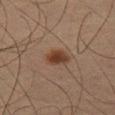Case summary:
- biopsy status · no biopsy performed (imaged during a skin exam)
- automated lesion analysis · a footprint of about 5 mm², a shape eccentricity near 0.65, and two-axis asymmetry of about 0.25; a border-irregularity rating of about 2/10, a color-variation rating of about 3.5/10, and peripheral color asymmetry of about 1; an automated nevus-likeness rating near 100 out of 100 and a lesion-detection confidence of about 100/100
- image · ~15 mm crop, total-body skin-cancer survey
- diameter · ~3 mm (longest diameter)
- patient · male, aged around 65
- anatomic site · the left thigh
- illumination · cross-polarized illumination